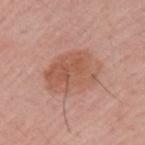{
  "biopsy_status": "not biopsied; imaged during a skin examination",
  "image": {
    "source": "total-body photography crop",
    "field_of_view_mm": 15
  },
  "patient": {
    "sex": "female",
    "age_approx": 55
  },
  "site": "arm"
}